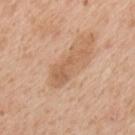<case>
<lesion_size>
  <long_diameter_mm_approx>6.0</long_diameter_mm_approx>
</lesion_size>
<lighting>white-light</lighting>
<patient>
  <sex>male</sex>
  <age_approx>65</age_approx>
</patient>
<site>upper back</site>
<automated_metrics>
  <eccentricity>0.95</eccentricity>
  <shape_asymmetry>0.45</shape_asymmetry>
  <cielab_L>60</cielab_L>
  <cielab_a>20</cielab_a>
  <cielab_b>34</cielab_b>
  <vs_skin_darker_L>8.0</vs_skin_darker_L>
  <vs_skin_contrast_norm>6.0</vs_skin_contrast_norm>
  <border_irregularity_0_10>5.5</border_irregularity_0_10>
  <color_variation_0_10>2.0</color_variation_0_10>
  <peripheral_color_asymmetry>0.5</peripheral_color_asymmetry>
  <lesion_detection_confidence_0_100>100</lesion_detection_confidence_0_100>
</automated_metrics>
<image>
  <source>total-body photography crop</source>
  <field_of_view_mm>15</field_of_view_mm>
</image>
</case>